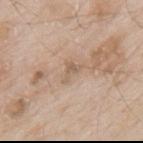Captured during whole-body skin photography for melanoma surveillance; the lesion was not biopsied.
A roughly 15 mm field-of-view crop from a total-body skin photograph.
An algorithmic analysis of the crop reported a lesion color around L≈59 a*≈14 b*≈29 in CIELAB and a normalized border contrast of about 5.5. It also reported a classifier nevus-likeness of about 0/100 and a detector confidence of about 95 out of 100 that the crop contains a lesion.
Longest diameter approximately 3 mm.
A male patient, in their mid-60s.
From the mid back.